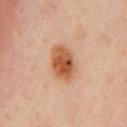follow-up: catalogued during a skin exam; not biopsied
image: ~15 mm crop, total-body skin-cancer survey
patient: male, aged around 65
tile lighting: cross-polarized illumination
location: the chest